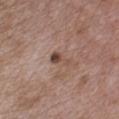No biopsy was performed on this lesion — it was imaged during a full skin examination and was not determined to be concerning.
From the chest.
The recorded lesion diameter is about 3 mm.
Automated image analysis of the tile measured a lesion–skin lightness drop of about 11 and a lesion-to-skin contrast of about 8 (normalized; higher = more distinct). And it measured a border-irregularity index near 6/10.
The patient is a male aged 48–52.
A close-up tile cropped from a whole-body skin photograph, about 15 mm across.